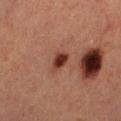Q: Was a biopsy performed?
A: no biopsy performed (imaged during a skin exam)
Q: How was the tile lit?
A: cross-polarized
Q: Automated lesion metrics?
A: an average lesion color of about L≈29 a*≈23 b*≈25 (CIELAB), roughly 13 lightness units darker than nearby skin, and a normalized border contrast of about 12; a classifier nevus-likeness of about 100/100
Q: What is the lesion's diameter?
A: ~2 mm (longest diameter)
Q: What is the anatomic site?
A: the leg
Q: How was this image acquired?
A: 15 mm crop, total-body photography
Q: Who is the patient?
A: female, aged 38 to 42